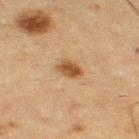Impression: Recorded during total-body skin imaging; not selected for excision or biopsy. Context: The total-body-photography lesion software estimated a lesion area of about 5 mm², an eccentricity of roughly 0.7, and two-axis asymmetry of about 0.2. And it measured a border-irregularity rating of about 2/10, a color-variation rating of about 3.5/10, and peripheral color asymmetry of about 1.5. A lesion tile, about 15 mm wide, cut from a 3D total-body photograph. A male subject, in their mid- to late 60s. The lesion's longest dimension is about 3 mm. The tile uses cross-polarized illumination. Located on the left thigh.